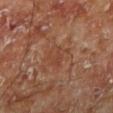- biopsy status: catalogued during a skin exam; not biopsied
- anatomic site: the left lower leg
- acquisition: ~15 mm tile from a whole-body skin photo
- patient: male, aged around 70